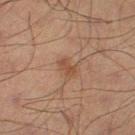Q: Was a biopsy performed?
A: imaged on a skin check; not biopsied
Q: What are the patient's age and sex?
A: male, aged approximately 70
Q: How was the tile lit?
A: cross-polarized illumination
Q: Lesion location?
A: the leg
Q: How was this image acquired?
A: total-body-photography crop, ~15 mm field of view
Q: How large is the lesion?
A: ~2.5 mm (longest diameter)